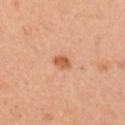{"biopsy_status": "not biopsied; imaged during a skin examination", "lesion_size": {"long_diameter_mm_approx": 2.0}, "site": "arm", "patient": {"sex": "female", "age_approx": 30}, "automated_metrics": {"area_mm2_approx": 3.0, "eccentricity": 0.65, "shape_asymmetry": 0.25, "cielab_L": 55, "cielab_a": 25, "cielab_b": 37, "vs_skin_darker_L": 11.0, "vs_skin_contrast_norm": 8.5, "border_irregularity_0_10": 2.0, "color_variation_0_10": 2.0, "peripheral_color_asymmetry": 0.5}, "image": {"source": "total-body photography crop", "field_of_view_mm": 15}}